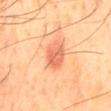biopsy_status: not biopsied; imaged during a skin examination
lighting: cross-polarized
image:
  source: total-body photography crop
  field_of_view_mm: 15
site: mid back
patient:
  sex: male
  age_approx: 45
automated_metrics:
  eccentricity: 0.7
  cielab_L: 65
  cielab_a: 31
  cielab_b: 38
  vs_skin_contrast_norm: 6.5